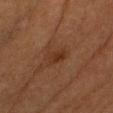– notes: total-body-photography surveillance lesion; no biopsy
– anatomic site: the front of the torso
– subject: female, aged around 55
– image source: 15 mm crop, total-body photography